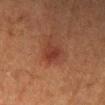• location — the right lower leg
• imaging modality — 15 mm crop, total-body photography
• subject — female, in their 50s
• automated lesion analysis — an area of roughly 5.5 mm², a shape eccentricity near 0.5, and two-axis asymmetry of about 0.3; a border-irregularity index near 3/10, a within-lesion color-variation index near 2.5/10, and a peripheral color-asymmetry measure near 1; an automated nevus-likeness rating near 55 out of 100 and a detector confidence of about 100 out of 100 that the crop contains a lesion
• diameter — ~3 mm (longest diameter)
• tile lighting — cross-polarized illumination